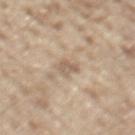Clinical impression:
Imaged during a routine full-body skin examination; the lesion was not biopsied and no histopathology is available.
Context:
The lesion is located on the mid back. Longest diameter approximately 2.5 mm. Captured under white-light illumination. A region of skin cropped from a whole-body photographic capture, roughly 15 mm wide. A male patient, approximately 70 years of age.